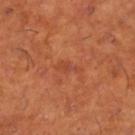Q: Is there a histopathology result?
A: imaged on a skin check; not biopsied
Q: Patient demographics?
A: male, aged approximately 65
Q: What lighting was used for the tile?
A: cross-polarized illumination
Q: Automated lesion metrics?
A: an average lesion color of about L≈46 a*≈30 b*≈36 (CIELAB), roughly 6 lightness units darker than nearby skin, and a normalized lesion–skin contrast near 4.5; a border-irregularity rating of about 5/10 and radial color variation of about 0
Q: What is the lesion's diameter?
A: about 3 mm
Q: Lesion location?
A: the right lower leg
Q: What is the imaging modality?
A: ~15 mm crop, total-body skin-cancer survey